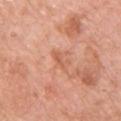Clinical impression: The lesion was tiled from a total-body skin photograph and was not biopsied. Clinical summary: The lesion is located on the chest. Automated tile analysis of the lesion measured an average lesion color of about L≈60 a*≈27 b*≈34 (CIELAB) and roughly 8 lightness units darker than nearby skin. The analysis additionally found a within-lesion color-variation index near 0/10 and a peripheral color-asymmetry measure near 0. Cropped from a whole-body photographic skin survey; the tile spans about 15 mm. The tile uses white-light illumination. The subject is a male aged 53–57. About 2.5 mm across.